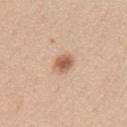notes = imaged on a skin check; not biopsied
site = the mid back
lesion diameter = ~2.5 mm (longest diameter)
illumination = white-light illumination
image source = total-body-photography crop, ~15 mm field of view
patient = female, roughly 40 years of age